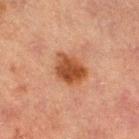workup: no biopsy performed (imaged during a skin exam) | site: the left thigh | lighting: cross-polarized illumination | patient: male, about 65 years old | image source: ~15 mm tile from a whole-body skin photo.